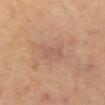The lesion was tiled from a total-body skin photograph and was not biopsied. This image is a 15 mm lesion crop taken from a total-body photograph. On the abdomen. Captured under cross-polarized illumination. Measured at roughly 2.5 mm in maximum diameter. The patient is a male aged approximately 65.